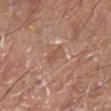<case>
  <biopsy_status>not biopsied; imaged during a skin examination</biopsy_status>
  <image>
    <source>total-body photography crop</source>
    <field_of_view_mm>15</field_of_view_mm>
  </image>
  <patient>
    <sex>male</sex>
    <age_approx>70</age_approx>
  </patient>
  <site>left lower leg</site>
</case>